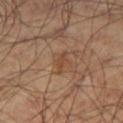Q: Is there a histopathology result?
A: no biopsy performed (imaged during a skin exam)
Q: How large is the lesion?
A: about 2.5 mm
Q: What is the imaging modality?
A: ~15 mm crop, total-body skin-cancer survey
Q: What is the anatomic site?
A: the leg
Q: What are the patient's age and sex?
A: male, about 60 years old
Q: Illumination type?
A: cross-polarized